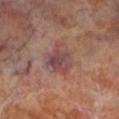Q: Is there a histopathology result?
A: catalogued during a skin exam; not biopsied
Q: Where on the body is the lesion?
A: the left lower leg
Q: How was this image acquired?
A: ~15 mm crop, total-body skin-cancer survey
Q: Illumination type?
A: cross-polarized
Q: Patient demographics?
A: male, about 70 years old
Q: What is the lesion's diameter?
A: ≈3.5 mm